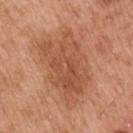workup = total-body-photography surveillance lesion; no biopsy
lighting = white-light
body site = the right upper arm
acquisition = total-body-photography crop, ~15 mm field of view
lesion diameter = ~8.5 mm (longest diameter)
subject = male, approximately 55 years of age